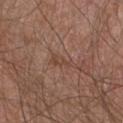follow-up: imaged on a skin check; not biopsied | acquisition: ~15 mm crop, total-body skin-cancer survey | location: the front of the torso | patient: male, aged 63 to 67.